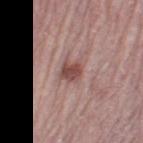image: ~15 mm tile from a whole-body skin photo
patient: female, in their mid-60s
illumination: white-light
size: ~2.5 mm (longest diameter)
body site: the left thigh
image-analysis metrics: a lesion area of about 5 mm², an eccentricity of roughly 0.2, and a shape-asymmetry score of about 0.25 (0 = symmetric); a lesion color around L≈48 a*≈22 b*≈22 in CIELAB and a lesion-to-skin contrast of about 8.5 (normalized; higher = more distinct)